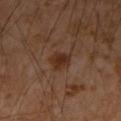The lesion was photographed on a routine skin check and not biopsied; there is no pathology result.
On the right forearm.
The tile uses cross-polarized illumination.
The lesion's longest dimension is about 2.5 mm.
A male patient aged approximately 55.
A lesion tile, about 15 mm wide, cut from a 3D total-body photograph.